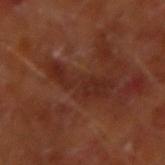Findings:
• workup · catalogued during a skin exam; not biopsied
• subject · male, in their mid-60s
• site · the right forearm
• image source · 15 mm crop, total-body photography